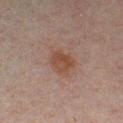biopsy_status: not biopsied; imaged during a skin examination
patient:
  sex: male
  age_approx: 30
image:
  source: total-body photography crop
  field_of_view_mm: 15
lesion_size:
  long_diameter_mm_approx: 3.5
site: chest